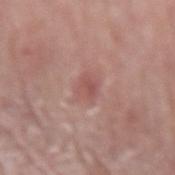workup: imaged on a skin check; not biopsied
tile lighting: white-light
subject: male, aged approximately 70
automated lesion analysis: a footprint of about 4.5 mm², an outline eccentricity of about 0.7 (0 = round, 1 = elongated), and two-axis asymmetry of about 0.25; border irregularity of about 2 on a 0–10 scale, internal color variation of about 2.5 on a 0–10 scale, and radial color variation of about 1; lesion-presence confidence of about 100/100
image: 15 mm crop, total-body photography
diameter: about 3 mm
site: the left forearm